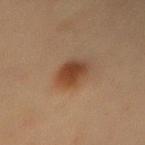Assessment:
Captured during whole-body skin photography for melanoma surveillance; the lesion was not biopsied.
Clinical summary:
Cropped from a total-body skin-imaging series; the visible field is about 15 mm. Located on the left forearm. A female subject aged 38–42.